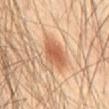Recorded during total-body skin imaging; not selected for excision or biopsy.
From the abdomen.
A roughly 15 mm field-of-view crop from a total-body skin photograph.
Longest diameter approximately 4 mm.
A male subject, aged 58–62.
The tile uses cross-polarized illumination.